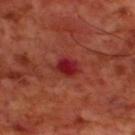  biopsy_status: not biopsied; imaged during a skin examination
  site: back
  lighting: cross-polarized
  image:
    source: total-body photography crop
    field_of_view_mm: 15
  patient:
    sex: male
    age_approx: 70